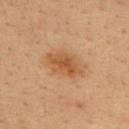Q: Was a biopsy performed?
A: catalogued during a skin exam; not biopsied
Q: How large is the lesion?
A: about 5 mm
Q: Patient demographics?
A: male, aged 33–37
Q: How was the tile lit?
A: cross-polarized illumination
Q: What did automated image analysis measure?
A: a lesion area of about 12 mm², a shape eccentricity near 0.8, and a shape-asymmetry score of about 0.2 (0 = symmetric); a classifier nevus-likeness of about 70/100 and a lesion-detection confidence of about 100/100
Q: Where on the body is the lesion?
A: the back
Q: How was this image acquired?
A: total-body-photography crop, ~15 mm field of view